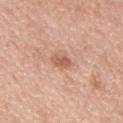No biopsy was performed on this lesion — it was imaged during a full skin examination and was not determined to be concerning.
Approximately 2.5 mm at its widest.
Imaged with white-light lighting.
A 15 mm crop from a total-body photograph taken for skin-cancer surveillance.
A male patient aged approximately 50.
On the mid back.
The total-body-photography lesion software estimated a lesion area of about 4 mm² and a symmetry-axis asymmetry near 0.25. And it measured a border-irregularity index near 2/10, a within-lesion color-variation index near 2.5/10, and peripheral color asymmetry of about 0.5. It also reported lesion-presence confidence of about 100/100.